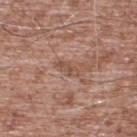  biopsy_status: not biopsied; imaged during a skin examination
  image:
    source: total-body photography crop
    field_of_view_mm: 15
  patient:
    sex: male
    age_approx: 55
  site: upper back